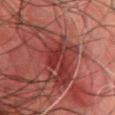biopsy_status: not biopsied; imaged during a skin examination
patient:
  sex: male
  age_approx: 70
site: chest
image:
  source: total-body photography crop
  field_of_view_mm: 15